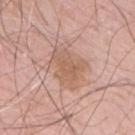{
  "biopsy_status": "not biopsied; imaged during a skin examination",
  "patient": {
    "sex": "male",
    "age_approx": 60
  },
  "image": {
    "source": "total-body photography crop",
    "field_of_view_mm": 15
  },
  "site": "mid back"
}